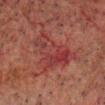notes = imaged on a skin check; not biopsied | diameter = ~7 mm (longest diameter) | image = 15 mm crop, total-body photography | location = the head or neck | tile lighting = cross-polarized illumination | subject = male, aged 58 to 62.